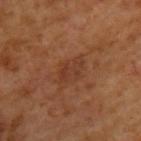A 15 mm close-up extracted from a 3D total-body photography capture.
The lesion is located on the upper back.
Longest diameter approximately 3.5 mm.
The patient is a male aged 63–67.
Imaged with cross-polarized lighting.